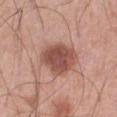<case>
<biopsy_status>not biopsied; imaged during a skin examination</biopsy_status>
<site>abdomen</site>
<image>
  <source>total-body photography crop</source>
  <field_of_view_mm>15</field_of_view_mm>
</image>
<patient>
  <sex>male</sex>
  <age_approx>70</age_approx>
</patient>
</case>